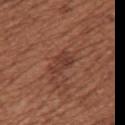– notes: total-body-photography surveillance lesion; no biopsy
– acquisition: ~15 mm crop, total-body skin-cancer survey
– site: the upper back
– subject: female, approximately 65 years of age
– lesion size: about 3.5 mm
– illumination: white-light illumination